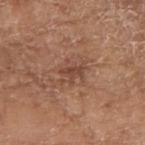{
  "biopsy_status": "not biopsied; imaged during a skin examination",
  "image": {
    "source": "total-body photography crop",
    "field_of_view_mm": 15
  },
  "site": "left forearm",
  "lighting": "white-light",
  "patient": {
    "sex": "female",
    "age_approx": 75
  },
  "lesion_size": {
    "long_diameter_mm_approx": 3.0
  }
}